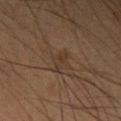Imaged during a routine full-body skin examination; the lesion was not biopsied and no histopathology is available.
About 3.5 mm across.
Imaged with cross-polarized lighting.
Cropped from a whole-body photographic skin survey; the tile spans about 15 mm.
A male subject in their mid- to late 50s.
On the right upper arm.